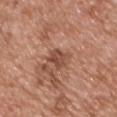Captured during whole-body skin photography for melanoma surveillance; the lesion was not biopsied. The tile uses white-light illumination. Measured at roughly 3 mm in maximum diameter. A male patient aged 48–52. From the chest. A 15 mm close-up tile from a total-body photography series done for melanoma screening.